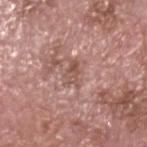Q: Is there a histopathology result?
A: catalogued during a skin exam; not biopsied
Q: Patient demographics?
A: male, roughly 75 years of age
Q: What lighting was used for the tile?
A: white-light
Q: What did automated image analysis measure?
A: a border-irregularity rating of about 4/10 and internal color variation of about 5 on a 0–10 scale
Q: What is the anatomic site?
A: the head or neck
Q: What is the imaging modality?
A: 15 mm crop, total-body photography